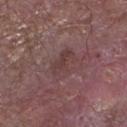Q: Is there a histopathology result?
A: imaged on a skin check; not biopsied
Q: Patient demographics?
A: male, aged 78–82
Q: What is the anatomic site?
A: the left lower leg
Q: How was the tile lit?
A: white-light
Q: What is the imaging modality?
A: total-body-photography crop, ~15 mm field of view